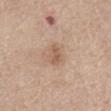follow-up = catalogued during a skin exam; not biopsied | lighting = white-light illumination | image-analysis metrics = an average lesion color of about L≈58 a*≈19 b*≈30 (CIELAB), about 9 CIELAB-L* units darker than the surrounding skin, and a normalized border contrast of about 6.5; border irregularity of about 2.5 on a 0–10 scale and a within-lesion color-variation index near 2/10 | patient = female, approximately 65 years of age | image source = 15 mm crop, total-body photography | body site = the abdomen.